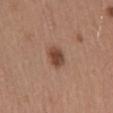Captured during whole-body skin photography for melanoma surveillance; the lesion was not biopsied. Captured under white-light illumination. The lesion-visualizer software estimated an average lesion color of about L≈45 a*≈21 b*≈28 (CIELAB), roughly 12 lightness units darker than nearby skin, and a normalized lesion–skin contrast near 9. And it measured a border-irregularity rating of about 1.5/10 and a peripheral color-asymmetry measure near 1. Located on the mid back. Approximately 3 mm at its widest. A close-up tile cropped from a whole-body skin photograph, about 15 mm across. A male patient, aged approximately 55.